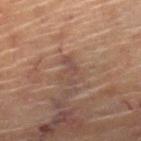Case summary:
- biopsy status · no biopsy performed (imaged during a skin exam)
- tile lighting · cross-polarized illumination
- subject · male, about 85 years old or older
- automated metrics · a footprint of about 3.5 mm², an eccentricity of roughly 0.9, and a shape-asymmetry score of about 0.55 (0 = symmetric); an average lesion color of about L≈40 a*≈16 b*≈21 (CIELAB), about 6 CIELAB-L* units darker than the surrounding skin, and a lesion-to-skin contrast of about 5.5 (normalized; higher = more distinct); a border-irregularity index near 7.5/10, internal color variation of about 0 on a 0–10 scale, and a peripheral color-asymmetry measure near 0
- acquisition · ~15 mm crop, total-body skin-cancer survey
- size · ~3.5 mm (longest diameter)
- body site · the leg